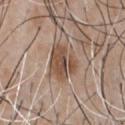Captured during whole-body skin photography for melanoma surveillance; the lesion was not biopsied. Longest diameter approximately 4.5 mm. Located on the chest. The subject is a male in their 50s. A close-up tile cropped from a whole-body skin photograph, about 15 mm across. This is a white-light tile.